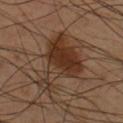biopsy_status: not biopsied; imaged during a skin examination
lesion_size:
  long_diameter_mm_approx: 7.5
image:
  source: total-body photography crop
  field_of_view_mm: 15
patient:
  sex: male
  age_approx: 40
site: front of the torso